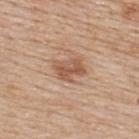Clinical summary:
The lesion is located on the upper back. A male subject about 60 years old. A 15 mm close-up extracted from a 3D total-body photography capture.